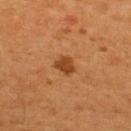Captured during whole-body skin photography for melanoma surveillance; the lesion was not biopsied. Located on the back. A lesion tile, about 15 mm wide, cut from a 3D total-body photograph. Imaged with cross-polarized lighting. The patient is a male roughly 55 years of age. Automated tile analysis of the lesion measured a lesion area of about 4.5 mm² and two-axis asymmetry of about 0.25. The analysis additionally found a lesion–skin lightness drop of about 10 and a lesion-to-skin contrast of about 8.5 (normalized; higher = more distinct). It also reported a border-irregularity index near 2.5/10, internal color variation of about 1.5 on a 0–10 scale, and radial color variation of about 0.5. The software also gave a detector confidence of about 100 out of 100 that the crop contains a lesion. The recorded lesion diameter is about 2.5 mm.